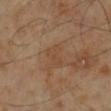notes: imaged on a skin check; not biopsied | lighting: cross-polarized | acquisition: ~15 mm crop, total-body skin-cancer survey | TBP lesion metrics: an area of roughly 5 mm², a shape eccentricity near 0.8, and a shape-asymmetry score of about 0.4 (0 = symmetric); an average lesion color of about L≈44 a*≈18 b*≈31 (CIELAB) and a lesion–skin lightness drop of about 5 | patient: male, aged around 70 | lesion size: ~3.5 mm (longest diameter) | body site: the leg.